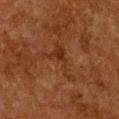follow-up: total-body-photography surveillance lesion; no biopsy | automated lesion analysis: a border-irregularity index near 10/10, internal color variation of about 1.5 on a 0–10 scale, and radial color variation of about 0.5 | image: ~15 mm crop, total-body skin-cancer survey | anatomic site: the chest | tile lighting: cross-polarized | lesion diameter: about 5.5 mm | patient: female, aged approximately 50.